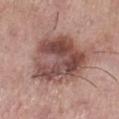Assessment:
No biopsy was performed on this lesion — it was imaged during a full skin examination and was not determined to be concerning.
Background:
Imaged with white-light lighting. The total-body-photography lesion software estimated an area of roughly 35 mm², an outline eccentricity of about 0.7 (0 = round, 1 = elongated), and two-axis asymmetry of about 0.25. The software also gave an average lesion color of about L≈49 a*≈21 b*≈23 (CIELAB), a lesion–skin lightness drop of about 14, and a normalized lesion–skin contrast near 10. The analysis additionally found a classifier nevus-likeness of about 35/100 and a detector confidence of about 100 out of 100 that the crop contains a lesion. The lesion is located on the right lower leg. A roughly 15 mm field-of-view crop from a total-body skin photograph. A female patient, about 70 years old.